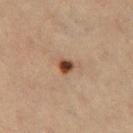Imaged during a routine full-body skin examination; the lesion was not biopsied and no histopathology is available.
On the left thigh.
A female patient in their mid- to late 60s.
A 15 mm close-up tile from a total-body photography series done for melanoma screening.
The recorded lesion diameter is about 2 mm.
Captured under cross-polarized illumination.
Automated image analysis of the tile measured an area of roughly 2.5 mm², a shape eccentricity near 0.4, and a symmetry-axis asymmetry near 0.25. It also reported a lesion–skin lightness drop of about 15 and a normalized border contrast of about 12.5. The software also gave a border-irregularity rating of about 2/10 and radial color variation of about 1.5.